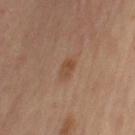Case summary:
• biopsy status — catalogued during a skin exam; not biopsied
• site — the left upper arm
• illumination — cross-polarized
• acquisition — ~15 mm tile from a whole-body skin photo
• subject — female, about 65 years old
• lesion size — ≈2 mm